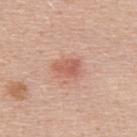  biopsy_status: not biopsied; imaged during a skin examination
  lesion_size:
    long_diameter_mm_approx: 3.0
  patient:
    sex: male
    age_approx: 40
  site: upper back
  lighting: white-light
  image:
    source: total-body photography crop
    field_of_view_mm: 15